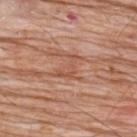notes = catalogued during a skin exam; not biopsied
size = about 3.5 mm
subject = male, in their 80s
lighting = white-light illumination
image = 15 mm crop, total-body photography
automated lesion analysis = a lesion area of about 4 mm² and two-axis asymmetry of about 0.7; an automated nevus-likeness rating near 0 out of 100 and a lesion-detection confidence of about 65/100
site = the upper back